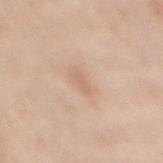Q: Was a biopsy performed?
A: no biopsy performed (imaged during a skin exam)
Q: Illumination type?
A: white-light
Q: Lesion location?
A: the mid back
Q: Patient demographics?
A: female, approximately 35 years of age
Q: What is the imaging modality?
A: 15 mm crop, total-body photography
Q: Lesion size?
A: ~2.5 mm (longest diameter)